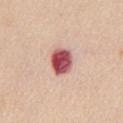{"patient": {"sex": "female", "age_approx": 55}, "image": {"source": "total-body photography crop", "field_of_view_mm": 15}, "lighting": "white-light", "automated_metrics": {"eccentricity": 0.7, "shape_asymmetry": 0.15, "cielab_L": 54, "cielab_a": 32, "cielab_b": 23, "vs_skin_darker_L": 21.0, "nevus_likeness_0_100": 0}, "site": "chest"}